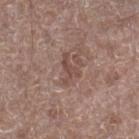Assessment:
Captured during whole-body skin photography for melanoma surveillance; the lesion was not biopsied.
Acquisition and patient details:
A 15 mm close-up tile from a total-body photography series done for melanoma screening. A male subject, in their 70s. The lesion is located on the right lower leg. The recorded lesion diameter is about 3.5 mm. Automated image analysis of the tile measured a normalized border contrast of about 6. It also reported border irregularity of about 8.5 on a 0–10 scale, a color-variation rating of about 0/10, and a peripheral color-asymmetry measure near 0. The tile uses white-light illumination.